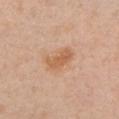<record>
<biopsy_status>not biopsied; imaged during a skin examination</biopsy_status>
<site>chest</site>
<lighting>white-light</lighting>
<patient>
  <sex>male</sex>
  <age_approx>55</age_approx>
</patient>
<automated_metrics>
  <area_mm2_approx>7.0</area_mm2_approx>
  <eccentricity>0.85</eccentricity>
  <shape_asymmetry>0.25</shape_asymmetry>
  <cielab_L>61</cielab_L>
  <cielab_a>21</cielab_a>
  <cielab_b>35</cielab_b>
  <vs_skin_darker_L>9.0</vs_skin_darker_L>
  <border_irregularity_0_10>2.5</border_irregularity_0_10>
  <color_variation_0_10>2.5</color_variation_0_10>
  <peripheral_color_asymmetry>1.0</peripheral_color_asymmetry>
  <nevus_likeness_0_100>45</nevus_likeness_0_100>
  <lesion_detection_confidence_0_100>100</lesion_detection_confidence_0_100>
</automated_metrics>
<image>
  <source>total-body photography crop</source>
  <field_of_view_mm>15</field_of_view_mm>
</image>
</record>